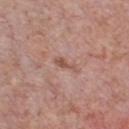follow-up — imaged on a skin check; not biopsied | image-analysis metrics — a footprint of about 2.5 mm², a shape eccentricity near 0.95, and two-axis asymmetry of about 0.4; roughly 9 lightness units darker than nearby skin and a normalized border contrast of about 6.5; border irregularity of about 4.5 on a 0–10 scale, a color-variation rating of about 0.5/10, and peripheral color asymmetry of about 0 | illumination — white-light illumination | body site — the chest | patient — male, roughly 60 years of age | image source — ~15 mm tile from a whole-body skin photo | lesion size — ~3 mm (longest diameter).